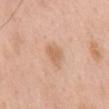Q: Is there a histopathology result?
A: no biopsy performed (imaged during a skin exam)
Q: How was this image acquired?
A: ~15 mm tile from a whole-body skin photo
Q: What lighting was used for the tile?
A: white-light
Q: Patient demographics?
A: male, about 60 years old
Q: Where on the body is the lesion?
A: the back